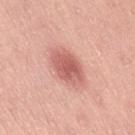No biopsy was performed on this lesion — it was imaged during a full skin examination and was not determined to be concerning.